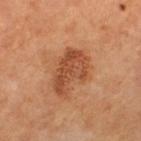<lesion>
  <biopsy_status>not biopsied; imaged during a skin examination</biopsy_status>
  <automated_metrics>
    <area_mm2_approx>13.0</area_mm2_approx>
    <eccentricity>0.8</eccentricity>
    <shape_asymmetry>0.35</shape_asymmetry>
    <cielab_L>48</cielab_L>
    <cielab_a>26</cielab_a>
    <cielab_b>35</cielab_b>
    <vs_skin_darker_L>11.0</vs_skin_darker_L>
    <vs_skin_contrast_norm>7.5</vs_skin_contrast_norm>
    <border_irregularity_0_10>4.5</border_irregularity_0_10>
    <color_variation_0_10>3.5</color_variation_0_10>
    <peripheral_color_asymmetry>1.0</peripheral_color_asymmetry>
  </automated_metrics>
  <patient>
    <age_approx>70</age_approx>
  </patient>
  <site>left thigh</site>
  <lesion_size>
    <long_diameter_mm_approx>5.5</long_diameter_mm_approx>
  </lesion_size>
  <lighting>cross-polarized</lighting>
  <image>
    <source>total-body photography crop</source>
    <field_of_view_mm>15</field_of_view_mm>
  </image>
</lesion>